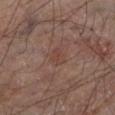This lesion was catalogued during total-body skin photography and was not selected for biopsy. A male patient, in their mid-60s. Automated tile analysis of the lesion measured a border-irregularity rating of about 5/10 and a peripheral color-asymmetry measure near 0. It also reported lesion-presence confidence of about 100/100. A region of skin cropped from a whole-body photographic capture, roughly 15 mm wide. On the left lower leg. The tile uses cross-polarized illumination. The recorded lesion diameter is about 2.5 mm.